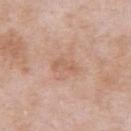Impression: The lesion was tiled from a total-body skin photograph and was not biopsied. Background: A region of skin cropped from a whole-body photographic capture, roughly 15 mm wide. The patient is a male about 55 years old. The total-body-photography lesion software estimated a lesion area of about 4 mm², a shape eccentricity near 0.9, and a shape-asymmetry score of about 0.45 (0 = symmetric). It also reported a lesion color around L≈60 a*≈21 b*≈31 in CIELAB, a lesion–skin lightness drop of about 7, and a normalized border contrast of about 5. Measured at roughly 3.5 mm in maximum diameter. From the left upper arm. Captured under white-light illumination.